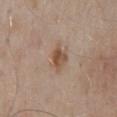biopsy status: imaged on a skin check; not biopsied | lesion size: about 3.5 mm | location: the back | patient: male, approximately 55 years of age | lighting: white-light illumination | automated metrics: an area of roughly 5.5 mm², a shape eccentricity near 0.7, and a symmetry-axis asymmetry near 0.25; a within-lesion color-variation index near 5/10 and peripheral color asymmetry of about 2 | image: total-body-photography crop, ~15 mm field of view.